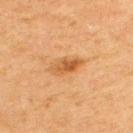Assessment:
This lesion was catalogued during total-body skin photography and was not selected for biopsy.
Image and clinical context:
A close-up tile cropped from a whole-body skin photograph, about 15 mm across. From the back. The subject is a male about 65 years old. The lesion's longest dimension is about 4 mm. Captured under cross-polarized illumination.